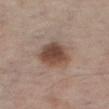Findings:
• workup — total-body-photography surveillance lesion; no biopsy
• location — the right thigh
• automated metrics — an average lesion color of about L≈46 a*≈18 b*≈25 (CIELAB) and roughly 13 lightness units darker than nearby skin; a peripheral color-asymmetry measure near 1.5; an automated nevus-likeness rating near 95 out of 100 and a lesion-detection confidence of about 100/100
• subject — male, aged approximately 75
• lesion diameter — about 4.5 mm
• imaging modality — 15 mm crop, total-body photography
• tile lighting — white-light illumination